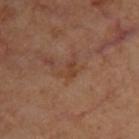No biopsy was performed on this lesion — it was imaged during a full skin examination and was not determined to be concerning.
On the upper back.
A 15 mm crop from a total-body photograph taken for skin-cancer surveillance.
A female subject in their mid- to late 40s.